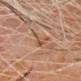This image is a 15 mm lesion crop taken from a total-body photograph.
This is a cross-polarized tile.
A male subject in their 80s.
The lesion is on the chest.